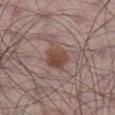Assessment:
The lesion was tiled from a total-body skin photograph and was not biopsied.
Image and clinical context:
The lesion is located on the right lower leg. A roughly 15 mm field-of-view crop from a total-body skin photograph. A male patient, aged 53 to 57. The recorded lesion diameter is about 3.5 mm. Automated image analysis of the tile measured a lesion area of about 7.5 mm² and an eccentricity of roughly 0.55. It also reported border irregularity of about 1.5 on a 0–10 scale, a color-variation rating of about 3.5/10, and a peripheral color-asymmetry measure near 1. The tile uses white-light illumination.